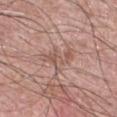site: chest
patient:
  sex: male
  age_approx: 55
image:
  source: total-body photography crop
  field_of_view_mm: 15